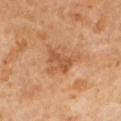Captured during whole-body skin photography for melanoma surveillance; the lesion was not biopsied. A roughly 15 mm field-of-view crop from a total-body skin photograph. A male patient, aged 63–67.